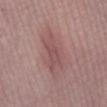Clinical impression: The lesion was photographed on a routine skin check and not biopsied; there is no pathology result. Context: A roughly 15 mm field-of-view crop from a total-body skin photograph. A female subject, aged around 60. From the left lower leg. Imaged with white-light lighting. Approximately 6 mm at its widest.